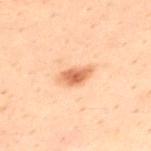<case>
  <biopsy_status>not biopsied; imaged during a skin examination</biopsy_status>
  <patient>
    <sex>male</sex>
    <age_approx>30</age_approx>
  </patient>
  <image>
    <source>total-body photography crop</source>
    <field_of_view_mm>15</field_of_view_mm>
  </image>
  <site>upper back</site>
  <lesion_size>
    <long_diameter_mm_approx>3.5</long_diameter_mm_approx>
  </lesion_size>
</case>